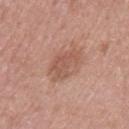No biopsy was performed on this lesion — it was imaged during a full skin examination and was not determined to be concerning. Located on the left upper arm. This image is a 15 mm lesion crop taken from a total-body photograph. A female subject, aged 48 to 52.